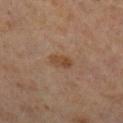workup: no biopsy performed (imaged during a skin exam) | patient: male, aged 58 to 62 | tile lighting: cross-polarized illumination | location: the right lower leg | size: about 2.5 mm | imaging modality: ~15 mm crop, total-body skin-cancer survey | image-analysis metrics: a lesion area of about 4 mm², a shape eccentricity near 0.8, and a symmetry-axis asymmetry near 0.25; a lesion–skin lightness drop of about 7 and a lesion-to-skin contrast of about 7 (normalized; higher = more distinct).